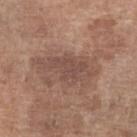| feature | finding |
|---|---|
| biopsy status | total-body-photography surveillance lesion; no biopsy |
| subject | female, aged around 80 |
| lesion diameter | ≈6.5 mm |
| image | total-body-photography crop, ~15 mm field of view |
| location | the left lower leg |
| TBP lesion metrics | a mean CIELAB color near L≈48 a*≈18 b*≈24 and a normalized border contrast of about 6 |